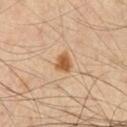A male subject in their mid- to late 30s.
Captured under cross-polarized illumination.
A lesion tile, about 15 mm wide, cut from a 3D total-body photograph.
Located on the chest.